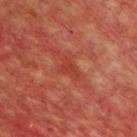A roughly 15 mm field-of-view crop from a total-body skin photograph. The total-body-photography lesion software estimated a lesion color around L≈40 a*≈33 b*≈32 in CIELAB, a lesion–skin lightness drop of about 6, and a normalized lesion–skin contrast near 5. It also reported border irregularity of about 4.5 on a 0–10 scale, internal color variation of about 1 on a 0–10 scale, and a peripheral color-asymmetry measure near 0.5. It also reported a nevus-likeness score of about 0/100 and a lesion-detection confidence of about 90/100. The recorded lesion diameter is about 3 mm. Located on the chest. The patient is a male aged 58 to 62.